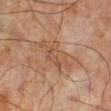imaging modality = ~15 mm tile from a whole-body skin photo; illumination = cross-polarized; anatomic site = the left lower leg; subject = male, in their mid-60s; size = about 5.5 mm.